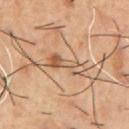The lesion was photographed on a routine skin check and not biopsied; there is no pathology result.
The lesion-visualizer software estimated a lesion area of about 7 mm², an outline eccentricity of about 0.95 (0 = round, 1 = elongated), and a shape-asymmetry score of about 0.4 (0 = symmetric). The software also gave a border-irregularity index near 5.5/10. And it measured a classifier nevus-likeness of about 10/100 and lesion-presence confidence of about 85/100.
Located on the chest.
Captured under cross-polarized illumination.
A male patient in their mid-50s.
This image is a 15 mm lesion crop taken from a total-body photograph.